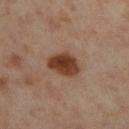Part of a total-body skin-imaging series; this lesion was reviewed on a skin check and was not flagged for biopsy. A 15 mm close-up extracted from a 3D total-body photography capture. A female patient, about 55 years old. On the right lower leg.